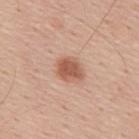Recorded during total-body skin imaging; not selected for excision or biopsy. Imaged with white-light lighting. A male patient approximately 55 years of age. The recorded lesion diameter is about 3.5 mm. From the upper back. The lesion-visualizer software estimated an area of roughly 6.5 mm², an eccentricity of roughly 0.6, and a symmetry-axis asymmetry near 0.2. The analysis additionally found a mean CIELAB color near L≈56 a*≈23 b*≈31, roughly 12 lightness units darker than nearby skin, and a normalized border contrast of about 8.5. The analysis additionally found a border-irregularity index near 2/10 and radial color variation of about 0.5. The software also gave a classifier nevus-likeness of about 100/100 and a detector confidence of about 100 out of 100 that the crop contains a lesion. Cropped from a total-body skin-imaging series; the visible field is about 15 mm.